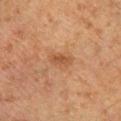{
  "biopsy_status": "not biopsied; imaged during a skin examination",
  "automated_metrics": {
    "vs_skin_darker_L": 8.0,
    "border_irregularity_0_10": 2.5,
    "color_variation_0_10": 2.5
  },
  "lesion_size": {
    "long_diameter_mm_approx": 3.0
  },
  "patient": {
    "sex": "male",
    "age_approx": 65
  },
  "lighting": "cross-polarized",
  "image": {
    "source": "total-body photography crop",
    "field_of_view_mm": 15
  },
  "site": "right lower leg"
}